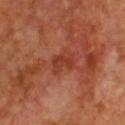This lesion was catalogued during total-body skin photography and was not selected for biopsy. The subject is a male in their mid-60s. Longest diameter approximately 2.5 mm. From the chest. Imaged with cross-polarized lighting. A 15 mm close-up extracted from a 3D total-body photography capture.